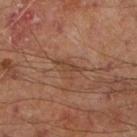Impression:
This lesion was catalogued during total-body skin photography and was not selected for biopsy.
Acquisition and patient details:
The tile uses cross-polarized illumination. The total-body-photography lesion software estimated an area of roughly 4.5 mm², an outline eccentricity of about 0.75 (0 = round, 1 = elongated), and a symmetry-axis asymmetry near 0.4. The software also gave a nevus-likeness score of about 0/100. Longest diameter approximately 3 mm. Cropped from a total-body skin-imaging series; the visible field is about 15 mm. A male patient, aged 63–67. The lesion is on the right lower leg.